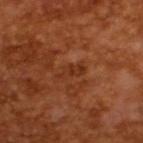biopsy_status: not biopsied; imaged during a skin examination
automated_metrics:
  shape_asymmetry: 0.35
  cielab_L: 32
  cielab_a: 25
  cielab_b: 33
  vs_skin_darker_L: 6.0
  vs_skin_contrast_norm: 6.0
  nevus_likeness_0_100: 0
  lesion_detection_confidence_0_100: 100
image:
  source: total-body photography crop
  field_of_view_mm: 15
lighting: cross-polarized
lesion_size:
  long_diameter_mm_approx: 3.5
patient:
  sex: male
  age_approx: 65